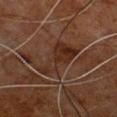<lesion>
  <biopsy_status>not biopsied; imaged during a skin examination</biopsy_status>
  <patient>
    <sex>male</sex>
    <age_approx>80</age_approx>
  </patient>
  <automated_metrics>
    <shape_asymmetry>0.45</shape_asymmetry>
    <border_irregularity_0_10>5.5</border_irregularity_0_10>
    <peripheral_color_asymmetry>1.5</peripheral_color_asymmetry>
  </automated_metrics>
  <site>front of the torso</site>
  <lighting>cross-polarized</lighting>
  <image>
    <source>total-body photography crop</source>
    <field_of_view_mm>15</field_of_view_mm>
  </image>
  <lesion_size>
    <long_diameter_mm_approx>5.5</long_diameter_mm_approx>
  </lesion_size>
</lesion>